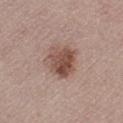– location · the left thigh
– imaging modality · total-body-photography crop, ~15 mm field of view
– patient · female, roughly 50 years of age